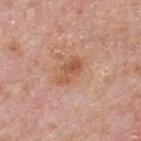Captured during whole-body skin photography for melanoma surveillance; the lesion was not biopsied. A male subject roughly 75 years of age. A 15 mm crop from a total-body photograph taken for skin-cancer surveillance. Located on the upper back.